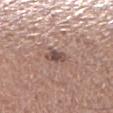• biopsy status — imaged on a skin check; not biopsied
• diameter — about 2.5 mm
• patient — female, approximately 50 years of age
• lighting — white-light
• automated lesion analysis — a mean CIELAB color near L≈48 a*≈18 b*≈21, a lesion–skin lightness drop of about 12, and a lesion-to-skin contrast of about 9 (normalized; higher = more distinct); a border-irregularity index near 2.5/10, a color-variation rating of about 2/10, and a peripheral color-asymmetry measure near 0.5
• body site — the right lower leg
• acquisition — ~15 mm tile from a whole-body skin photo